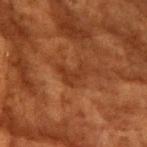subject — female, aged 78–82 | body site — the chest | acquisition — total-body-photography crop, ~15 mm field of view.